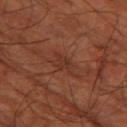Captured during whole-body skin photography for melanoma surveillance; the lesion was not biopsied. A patient about 65 years old. Longest diameter approximately 3 mm. The lesion is on the left thigh. Imaged with cross-polarized lighting. A roughly 15 mm field-of-view crop from a total-body skin photograph.